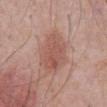Notes:
* workup — no biopsy performed (imaged during a skin exam)
* imaging modality — 15 mm crop, total-body photography
* lesion diameter — about 5.5 mm
* location — the abdomen
* illumination — white-light illumination
* subject — male, about 70 years old